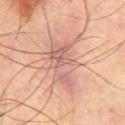image:
  source: total-body photography crop
  field_of_view_mm: 15
automated_metrics:
  area_mm2_approx: 15.0
  eccentricity: 0.85
  shape_asymmetry: 0.5
  lesion_detection_confidence_0_100: 85
site: mid back
patient:
  sex: male
  age_approx: 65
lesion_size:
  long_diameter_mm_approx: 7.0